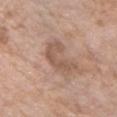Assessment: This lesion was catalogued during total-body skin photography and was not selected for biopsy. Clinical summary: Measured at roughly 4.5 mm in maximum diameter. A female patient aged around 75. This is a white-light tile. A 15 mm close-up extracted from a 3D total-body photography capture. On the chest.